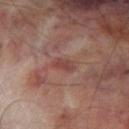<case>
<biopsy_status>not biopsied; imaged during a skin examination</biopsy_status>
<site>left thigh</site>
<patient>
  <sex>male</sex>
  <age_approx>70</age_approx>
</patient>
<automated_metrics>
  <area_mm2_approx>3.5</area_mm2_approx>
  <eccentricity>0.85</eccentricity>
  <shape_asymmetry>0.25</shape_asymmetry>
  <cielab_L>42</cielab_L>
  <cielab_a>23</cielab_a>
  <cielab_b>23</cielab_b>
  <vs_skin_darker_L>7.0</vs_skin_darker_L>
  <nevus_likeness_0_100>0</nevus_likeness_0_100>
</automated_metrics>
<lesion_size>
  <long_diameter_mm_approx>3.0</long_diameter_mm_approx>
</lesion_size>
<lighting>cross-polarized</lighting>
<image>
  <source>total-body photography crop</source>
  <field_of_view_mm>15</field_of_view_mm>
</image>
</case>